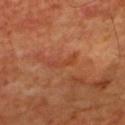| feature | finding |
|---|---|
| biopsy status | no biopsy performed (imaged during a skin exam) |
| subject | male, about 65 years old |
| image source | total-body-photography crop, ~15 mm field of view |
| body site | the upper back |
| image-analysis metrics | border irregularity of about 6 on a 0–10 scale and a peripheral color-asymmetry measure near 0; a nevus-likeness score of about 0/100 and lesion-presence confidence of about 100/100 |
| lighting | cross-polarized |